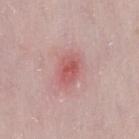Impression: Recorded during total-body skin imaging; not selected for excision or biopsy. Background: Captured under white-light illumination. Cropped from a total-body skin-imaging series; the visible field is about 15 mm. The lesion is on the right thigh. The patient is a female aged approximately 30. About 3 mm across.